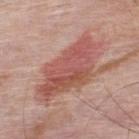notes = catalogued during a skin exam; not biopsied
patient = male, in their 50s
imaging modality = ~15 mm tile from a whole-body skin photo
lesion diameter = ~8 mm (longest diameter)
body site = the upper back
lighting = white-light illumination